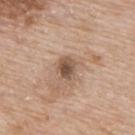{
  "biopsy_status": "not biopsied; imaged during a skin examination",
  "automated_metrics": {
    "area_mm2_approx": 4.5,
    "eccentricity": 0.65,
    "shape_asymmetry": 0.2,
    "border_irregularity_0_10": 2.0,
    "color_variation_0_10": 4.5,
    "peripheral_color_asymmetry": 1.5,
    "nevus_likeness_0_100": 20,
    "lesion_detection_confidence_0_100": 100
  },
  "site": "upper back",
  "lighting": "white-light",
  "patient": {
    "sex": "male",
    "age_approx": 65
  },
  "lesion_size": {
    "long_diameter_mm_approx": 2.5
  },
  "image": {
    "source": "total-body photography crop",
    "field_of_view_mm": 15
  }
}